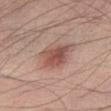| key | value |
|---|---|
| notes | imaged on a skin check; not biopsied |
| image | ~15 mm tile from a whole-body skin photo |
| body site | the abdomen |
| subject | male, aged 68–72 |